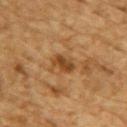biopsy_status: not biopsied; imaged during a skin examination
image:
  source: total-body photography crop
  field_of_view_mm: 15
site: upper back
patient:
  sex: male
  age_approx: 85+
lesion_size:
  long_diameter_mm_approx: 3.0